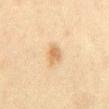Imaged during a routine full-body skin examination; the lesion was not biopsied and no histopathology is available.
A close-up tile cropped from a whole-body skin photograph, about 15 mm across.
Captured under cross-polarized illumination.
The subject is a female in their mid- to late 50s.
On the abdomen.
Automated image analysis of the tile measured an area of roughly 4.5 mm², an eccentricity of roughly 0.75, and a symmetry-axis asymmetry near 0.2. The software also gave a border-irregularity rating of about 2/10 and internal color variation of about 2 on a 0–10 scale. The analysis additionally found a classifier nevus-likeness of about 80/100 and lesion-presence confidence of about 100/100.